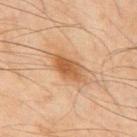Captured during whole-body skin photography for melanoma surveillance; the lesion was not biopsied.
Automated tile analysis of the lesion measured a mean CIELAB color near L≈47 a*≈18 b*≈32 and a normalized border contrast of about 8. The analysis additionally found an automated nevus-likeness rating near 90 out of 100 and a lesion-detection confidence of about 100/100.
The patient is a male in their mid-40s.
This image is a 15 mm lesion crop taken from a total-body photograph.
Approximately 5 mm at its widest.
On the mid back.